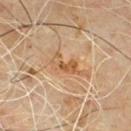Assessment:
Recorded during total-body skin imaging; not selected for excision or biopsy.
Background:
A close-up tile cropped from a whole-body skin photograph, about 15 mm across. Located on the back. A male patient, about 60 years old.